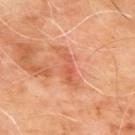{"biopsy_status": "not biopsied; imaged during a skin examination", "automated_metrics": {"area_mm2_approx": 6.0, "eccentricity": 0.95, "shape_asymmetry": 0.35, "border_irregularity_0_10": 5.0, "color_variation_0_10": 2.5, "peripheral_color_asymmetry": 0.5, "nevus_likeness_0_100": 0, "lesion_detection_confidence_0_100": 100}, "patient": {"sex": "male", "age_approx": 65}, "lighting": "cross-polarized", "lesion_size": {"long_diameter_mm_approx": 5.0}, "image": {"source": "total-body photography crop", "field_of_view_mm": 15}, "site": "back"}